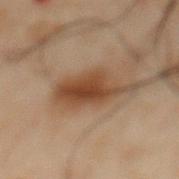Imaged during a routine full-body skin examination; the lesion was not biopsied and no histopathology is available.
A 15 mm close-up tile from a total-body photography series done for melanoma screening.
On the abdomen.
The subject is a male roughly 50 years of age.